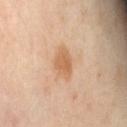Clinical impression: Recorded during total-body skin imaging; not selected for excision or biopsy. Image and clinical context: Measured at roughly 4 mm in maximum diameter. A roughly 15 mm field-of-view crop from a total-body skin photograph. Automated tile analysis of the lesion measured a mean CIELAB color near L≈61 a*≈20 b*≈35 and a lesion-to-skin contrast of about 6.5 (normalized; higher = more distinct). From the right thigh. The subject is a female aged 48 to 52.